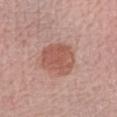| feature | finding |
|---|---|
| follow-up | total-body-photography surveillance lesion; no biopsy |
| automated metrics | a within-lesion color-variation index near 2.5/10 and a peripheral color-asymmetry measure near 1; a nevus-likeness score of about 90/100 |
| patient | female, in their mid-60s |
| location | the right forearm |
| lighting | white-light illumination |
| imaging modality | 15 mm crop, total-body photography |